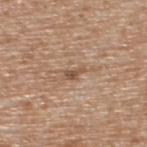This lesion was catalogued during total-body skin photography and was not selected for biopsy. This is a white-light tile. About 2.5 mm across. A male patient in their mid- to late 60s. A close-up tile cropped from a whole-body skin photograph, about 15 mm across. From the back.